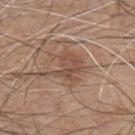Clinical summary: On the chest. Imaged with white-light lighting. The lesion-visualizer software estimated a footprint of about 10 mm² and a shape eccentricity near 0.65. The software also gave an automated nevus-likeness rating near 25 out of 100 and a detector confidence of about 95 out of 100 that the crop contains a lesion. Approximately 5.5 mm at its widest. A male patient, approximately 45 years of age. A roughly 15 mm field-of-view crop from a total-body skin photograph.